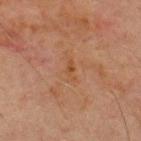The lesion was photographed on a routine skin check and not biopsied; there is no pathology result.
Approximately 2.5 mm at its widest.
A 15 mm close-up tile from a total-body photography series done for melanoma screening.
Automated image analysis of the tile measured about 5 CIELAB-L* units darker than the surrounding skin. It also reported internal color variation of about 0 on a 0–10 scale and radial color variation of about 0.
The lesion is on the chest.
A male subject aged around 70.